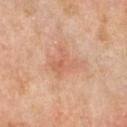Q: What are the patient's age and sex?
A: female, aged 68 to 72
Q: What lighting was used for the tile?
A: white-light illumination
Q: Lesion location?
A: the chest
Q: What kind of image is this?
A: total-body-photography crop, ~15 mm field of view